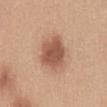Background: On the chest. Automated image analysis of the tile measured a footprint of about 13 mm², a shape eccentricity near 0.45, and a symmetry-axis asymmetry near 0.2. The analysis additionally found an average lesion color of about L≈54 a*≈22 b*≈30 (CIELAB), roughly 13 lightness units darker than nearby skin, and a normalized border contrast of about 9. The software also gave a border-irregularity rating of about 2/10 and a within-lesion color-variation index near 3/10. It also reported a nevus-likeness score of about 95/100. A roughly 15 mm field-of-view crop from a total-body skin photograph. The recorded lesion diameter is about 4.5 mm. A female patient, roughly 30 years of age.